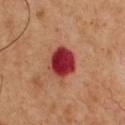No biopsy was performed on this lesion — it was imaged during a full skin examination and was not determined to be concerning.
Captured under cross-polarized illumination.
Cropped from a total-body skin-imaging series; the visible field is about 15 mm.
A male subject aged 48 to 52.
Longest diameter approximately 3.5 mm.
The lesion is on the chest.
Automated image analysis of the tile measured a footprint of about 11 mm² and a shape eccentricity near 0.45. It also reported an average lesion color of about L≈38 a*≈38 b*≈28 (CIELAB), a lesion–skin lightness drop of about 19, and a normalized lesion–skin contrast near 14.5.